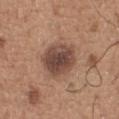Q: Was a biopsy performed?
A: catalogued during a skin exam; not biopsied
Q: What did automated image analysis measure?
A: a footprint of about 14 mm², an outline eccentricity of about 0.05 (0 = round, 1 = elongated), and a shape-asymmetry score of about 0.15 (0 = symmetric); a lesion color around L≈45 a*≈19 b*≈25 in CIELAB and roughly 13 lightness units darker than nearby skin; internal color variation of about 4.5 on a 0–10 scale and radial color variation of about 1; a nevus-likeness score of about 40/100 and lesion-presence confidence of about 100/100
Q: What is the lesion's diameter?
A: ~4.5 mm (longest diameter)
Q: Lesion location?
A: the front of the torso
Q: What are the patient's age and sex?
A: male, in their mid- to late 60s
Q: How was the tile lit?
A: white-light illumination
Q: How was this image acquired?
A: total-body-photography crop, ~15 mm field of view